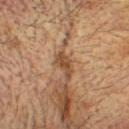Imaged during a routine full-body skin examination; the lesion was not biopsied and no histopathology is available.
The subject is a male in their mid- to late 70s.
The tile uses cross-polarized illumination.
The total-body-photography lesion software estimated a mean CIELAB color near L≈38 a*≈17 b*≈28, a lesion–skin lightness drop of about 10, and a normalized lesion–skin contrast near 8.5. The analysis additionally found a detector confidence of about 90 out of 100 that the crop contains a lesion.
The recorded lesion diameter is about 3 mm.
The lesion is located on the head or neck.
A 15 mm close-up extracted from a 3D total-body photography capture.